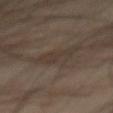The subject is a male aged 68 to 72.
The lesion is on the abdomen.
A 15 mm crop from a total-body photograph taken for skin-cancer surveillance.